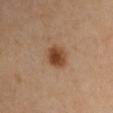This lesion was catalogued during total-body skin photography and was not selected for biopsy.
A male subject in their 40s.
Located on the chest.
A 15 mm crop from a total-body photograph taken for skin-cancer surveillance.
Captured under cross-polarized illumination.